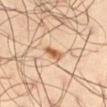The lesion was photographed on a routine skin check and not biopsied; there is no pathology result. This image is a 15 mm lesion crop taken from a total-body photograph. The lesion is located on the left thigh. The subject is a male approximately 60 years of age.